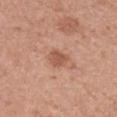workup — no biopsy performed (imaged during a skin exam) | tile lighting — white-light | acquisition — ~15 mm tile from a whole-body skin photo | diameter — ~2.5 mm (longest diameter) | patient — female, in their mid- to late 30s | body site — the left forearm | automated lesion analysis — a lesion area of about 4.5 mm², an eccentricity of roughly 0.45, and a symmetry-axis asymmetry near 0.2; a mean CIELAB color near L≈55 a*≈24 b*≈31, about 10 CIELAB-L* units darker than the surrounding skin, and a lesion-to-skin contrast of about 7 (normalized; higher = more distinct).